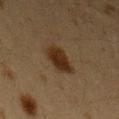– follow-up — no biopsy performed (imaged during a skin exam)